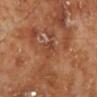biopsy status=imaged on a skin check; not biopsied | image=~15 mm crop, total-body skin-cancer survey | lighting=cross-polarized | patient=male, roughly 70 years of age | anatomic site=the left lower leg | automated lesion analysis=roughly 6 lightness units darker than nearby skin; a border-irregularity index near 3/10 and a peripheral color-asymmetry measure near 1.